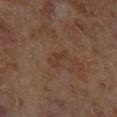{"biopsy_status": "not biopsied; imaged during a skin examination", "image": {"source": "total-body photography crop", "field_of_view_mm": 15}, "site": "left lower leg", "automated_metrics": {"area_mm2_approx": 3.0, "eccentricity": 0.9, "shape_asymmetry": 0.5}, "patient": {"sex": "male", "age_approx": 70}, "lesion_size": {"long_diameter_mm_approx": 3.0}}